The subject is a female about 40 years old. Captured under white-light illumination. From the back. A roughly 15 mm field-of-view crop from a total-body skin photograph: 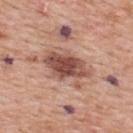The lesion was biopsied, and histopathology showed a dysplastic (Clark) nevus.